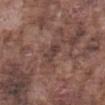Captured during whole-body skin photography for melanoma surveillance; the lesion was not biopsied.
A 15 mm close-up extracted from a 3D total-body photography capture.
Located on the abdomen.
The subject is a male in their mid- to late 70s.
This is a white-light tile.